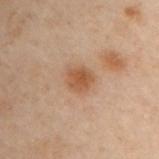A male patient in their 50s.
A 15 mm close-up extracted from a 3D total-body photography capture.
Located on the chest.